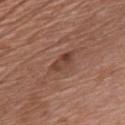biopsy status: imaged on a skin check; not biopsied | site: the chest | patient: male, aged 63–67 | automated metrics: a lesion color around L≈42 a*≈21 b*≈27 in CIELAB and roughly 9 lightness units darker than nearby skin; an automated nevus-likeness rating near 30 out of 100 and lesion-presence confidence of about 100/100 | size: ~3.5 mm (longest diameter) | image source: ~15 mm crop, total-body skin-cancer survey.